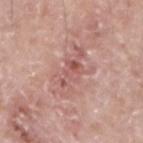Q: Is there a histopathology result?
A: no biopsy performed (imaged during a skin exam)
Q: Automated lesion metrics?
A: an area of roughly 13 mm² and a shape-asymmetry score of about 0.45 (0 = symmetric)
Q: How was this image acquired?
A: ~15 mm crop, total-body skin-cancer survey
Q: How was the tile lit?
A: white-light illumination
Q: Lesion location?
A: the right upper arm
Q: How large is the lesion?
A: ≈6.5 mm
Q: Who is the patient?
A: male, aged approximately 50